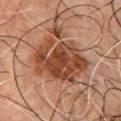The lesion was photographed on a routine skin check and not biopsied; there is no pathology result. A male subject, aged approximately 65. The lesion's longest dimension is about 7 mm. A 15 mm close-up extracted from a 3D total-body photography capture. The lesion is located on the chest. The tile uses cross-polarized illumination.